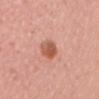The lesion was tiled from a total-body skin photograph and was not biopsied.
Longest diameter approximately 3 mm.
On the front of the torso.
This is a white-light tile.
A female subject, aged approximately 40.
Cropped from a total-body skin-imaging series; the visible field is about 15 mm.